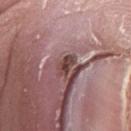  biopsy_status: not biopsied; imaged during a skin examination
  image:
    source: total-body photography crop
    field_of_view_mm: 15
  patient:
    sex: male
    age_approx: 45
  site: left forearm